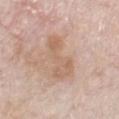The lesion is on the chest. The lesion's longest dimension is about 5.5 mm. This image is a 15 mm lesion crop taken from a total-body photograph. A male patient, roughly 75 years of age. The tile uses white-light illumination.